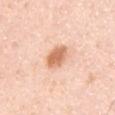• notes — imaged on a skin check; not biopsied
• body site — the chest
• automated lesion analysis — a border-irregularity rating of about 1/10 and a within-lesion color-variation index near 3/10
• imaging modality — total-body-photography crop, ~15 mm field of view
• tile lighting — white-light illumination
• subject — male, in their mid- to late 30s